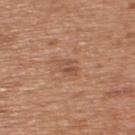{
  "biopsy_status": "not biopsied; imaged during a skin examination",
  "site": "upper back",
  "image": {
    "source": "total-body photography crop",
    "field_of_view_mm": 15
  },
  "patient": {
    "sex": "male",
    "age_approx": 65
  }
}